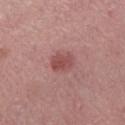<lesion>
  <image>
    <source>total-body photography crop</source>
    <field_of_view_mm>15</field_of_view_mm>
  </image>
  <lighting>white-light</lighting>
  <lesion_size>
    <long_diameter_mm_approx>3.0</long_diameter_mm_approx>
  </lesion_size>
  <patient>
    <sex>female</sex>
    <age_approx>50</age_approx>
  </patient>
  <site>left lower leg</site>
</lesion>